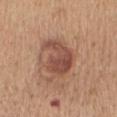Q: Is there a histopathology result?
A: imaged on a skin check; not biopsied
Q: Lesion size?
A: ≈5 mm
Q: Who is the patient?
A: female, aged 53 to 57
Q: What is the anatomic site?
A: the mid back
Q: How was this image acquired?
A: ~15 mm tile from a whole-body skin photo